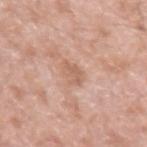| key | value |
|---|---|
| biopsy status | total-body-photography surveillance lesion; no biopsy |
| imaging modality | 15 mm crop, total-body photography |
| subject | male, aged 48–52 |
| lesion size | about 2.5 mm |
| tile lighting | white-light |
| location | the left upper arm |